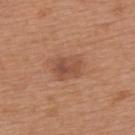Findings:
• workup — imaged on a skin check; not biopsied
• automated metrics — a shape eccentricity near 0.65; a border-irregularity rating of about 2/10 and peripheral color asymmetry of about 1.5
• location — the upper back
• diameter — about 3.5 mm
• imaging modality — ~15 mm crop, total-body skin-cancer survey
• illumination — white-light illumination
• subject — male, roughly 65 years of age